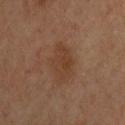<tbp_lesion>
<biopsy_status>not biopsied; imaged during a skin examination</biopsy_status>
<lesion_size>
  <long_diameter_mm_approx>4.5</long_diameter_mm_approx>
</lesion_size>
<image>
  <source>total-body photography crop</source>
  <field_of_view_mm>15</field_of_view_mm>
</image>
<patient>
  <sex>male</sex>
  <age_approx>35</age_approx>
</patient>
<lighting>cross-polarized</lighting>
<site>upper back</site>
</tbp_lesion>